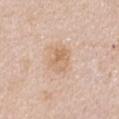<tbp_lesion>
  <biopsy_status>not biopsied; imaged during a skin examination</biopsy_status>
  <patient>
    <sex>female</sex>
    <age_approx>45</age_approx>
  </patient>
  <image>
    <source>total-body photography crop</source>
    <field_of_view_mm>15</field_of_view_mm>
  </image>
  <lighting>white-light</lighting>
  <lesion_size>
    <long_diameter_mm_approx>3.5</long_diameter_mm_approx>
  </lesion_size>
  <automated_metrics>
    <cielab_L>67</cielab_L>
    <cielab_a>17</cielab_a>
    <cielab_b>33</cielab_b>
    <vs_skin_contrast_norm>6.0</vs_skin_contrast_norm>
    <border_irregularity_0_10>2.0</border_irregularity_0_10>
    <color_variation_0_10>4.5</color_variation_0_10>
    <peripheral_color_asymmetry>1.5</peripheral_color_asymmetry>
  </automated_metrics>
  <site>front of the torso</site>
</tbp_lesion>